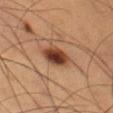This lesion was catalogued during total-body skin photography and was not selected for biopsy.
The subject is a male aged around 60.
The lesion's longest dimension is about 4 mm.
Cropped from a total-body skin-imaging series; the visible field is about 15 mm.
The total-body-photography lesion software estimated a footprint of about 8.5 mm², an outline eccentricity of about 0.8 (0 = round, 1 = elongated), and a shape-asymmetry score of about 0.2 (0 = symmetric). And it measured a border-irregularity index near 2/10, internal color variation of about 7 on a 0–10 scale, and radial color variation of about 2.
The tile uses cross-polarized illumination.
On the left thigh.